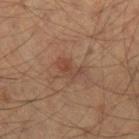Findings:
• notes · imaged on a skin check; not biopsied